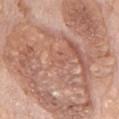| key | value |
|---|---|
| workup | total-body-photography surveillance lesion; no biopsy |
| subject | male, in their mid-70s |
| lesion size | ≈18 mm |
| image source | ~15 mm crop, total-body skin-cancer survey |
| automated lesion analysis | a footprint of about 125 mm² and a shape eccentricity near 0.7; an average lesion color of about L≈60 a*≈20 b*≈28 (CIELAB) and a normalized lesion–skin contrast near 7; a border-irregularity index near 8.5/10, a within-lesion color-variation index near 7.5/10, and peripheral color asymmetry of about 2.5 |
| location | the chest |